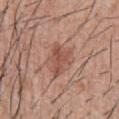Q: Was this lesion biopsied?
A: total-body-photography surveillance lesion; no biopsy
Q: How was this image acquired?
A: ~15 mm tile from a whole-body skin photo
Q: Patient demographics?
A: male, aged 53 to 57
Q: Lesion location?
A: the abdomen